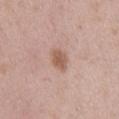{
  "biopsy_status": "not biopsied; imaged during a skin examination",
  "site": "mid back",
  "image": {
    "source": "total-body photography crop",
    "field_of_view_mm": 15
  },
  "lighting": "white-light",
  "lesion_size": {
    "long_diameter_mm_approx": 3.5
  },
  "automated_metrics": {
    "area_mm2_approx": 4.5,
    "eccentricity": 0.85,
    "shape_asymmetry": 0.2,
    "border_irregularity_0_10": 2.0,
    "color_variation_0_10": 1.5,
    "peripheral_color_asymmetry": 0.5,
    "nevus_likeness_0_100": 55,
    "lesion_detection_confidence_0_100": 100
  },
  "patient": {
    "sex": "female",
    "age_approx": 40
  }
}